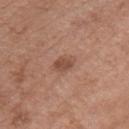Assessment: This lesion was catalogued during total-body skin photography and was not selected for biopsy. Acquisition and patient details: The lesion is located on the chest. Longest diameter approximately 2.5 mm. The subject is a male roughly 75 years of age. A 15 mm close-up extracted from a 3D total-body photography capture.